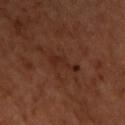{"biopsy_status": "not biopsied; imaged during a skin examination", "image": {"source": "total-body photography crop", "field_of_view_mm": 15}, "site": "head or neck", "lighting": "cross-polarized", "automated_metrics": {"eccentricity": 0.95, "shape_asymmetry": 0.55, "cielab_L": 27, "cielab_a": 22, "cielab_b": 26, "vs_skin_contrast_norm": 6.0, "nevus_likeness_0_100": 0, "lesion_detection_confidence_0_100": 100}, "patient": {"sex": "male", "age_approx": 65}, "lesion_size": {"long_diameter_mm_approx": 4.5}}